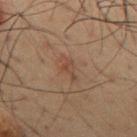A close-up tile cropped from a whole-body skin photograph, about 15 mm across. The lesion's longest dimension is about 3.5 mm. A male patient approximately 50 years of age. The lesion is located on the chest.